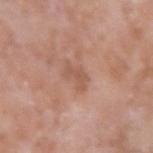Impression:
Part of a total-body skin-imaging series; this lesion was reviewed on a skin check and was not flagged for biopsy.
Background:
The subject is a male in their mid-70s. The lesion is on the right upper arm. The recorded lesion diameter is about 3 mm. A region of skin cropped from a whole-body photographic capture, roughly 15 mm wide. This is a white-light tile.